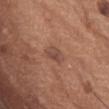<record>
  <biopsy_status>not biopsied; imaged during a skin examination</biopsy_status>
  <image>
    <source>total-body photography crop</source>
    <field_of_view_mm>15</field_of_view_mm>
  </image>
  <site>abdomen</site>
  <patient>
    <sex>female</sex>
    <age_approx>65</age_approx>
  </patient>
</record>